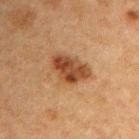workup — no biopsy performed (imaged during a skin exam)
lesion size — ~5 mm (longest diameter)
acquisition — 15 mm crop, total-body photography
tile lighting — cross-polarized illumination
anatomic site — the right upper arm
subject — male, aged 48–52